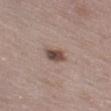The lesion was tiled from a total-body skin photograph and was not biopsied.
About 3 mm across.
A female subject, aged around 50.
A 15 mm close-up tile from a total-body photography series done for melanoma screening.
Captured under white-light illumination.
Located on the leg.